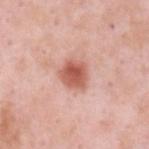Recorded during total-body skin imaging; not selected for excision or biopsy. Automated tile analysis of the lesion measured a lesion color around L≈58 a*≈28 b*≈29 in CIELAB, about 14 CIELAB-L* units darker than the surrounding skin, and a normalized border contrast of about 9. About 3.5 mm across. A male patient, aged around 35. A close-up tile cropped from a whole-body skin photograph, about 15 mm across. Located on the upper back.